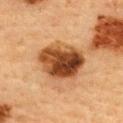Notes:
• workup — total-body-photography surveillance lesion; no biopsy
• imaging modality — 15 mm crop, total-body photography
• diameter — about 6 mm
• TBP lesion metrics — an area of roughly 21 mm² and an eccentricity of roughly 0.65; an average lesion color of about L≈42 a*≈23 b*≈35 (CIELAB), a lesion–skin lightness drop of about 18, and a normalized lesion–skin contrast near 13; border irregularity of about 2 on a 0–10 scale; a lesion-detection confidence of about 100/100
• patient — female, aged 58 to 62
• location — the upper back
• tile lighting — cross-polarized illumination